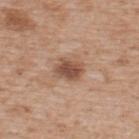Imaged during a routine full-body skin examination; the lesion was not biopsied and no histopathology is available. A region of skin cropped from a whole-body photographic capture, roughly 15 mm wide. A male patient about 65 years old. On the upper back.